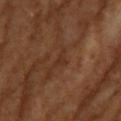Findings:
* biopsy status — no biopsy performed (imaged during a skin exam)
* image source — ~15 mm crop, total-body skin-cancer survey
* subject — female, aged 63–67
* lighting — cross-polarized illumination
* site — the upper back
* diameter — ≈2.5 mm